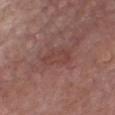Clinical impression: The lesion was tiled from a total-body skin photograph and was not biopsied. Clinical summary: From the front of the torso. This is a white-light tile. A roughly 15 mm field-of-view crop from a total-body skin photograph. The total-body-photography lesion software estimated two-axis asymmetry of about 0.25. The software also gave border irregularity of about 3 on a 0–10 scale and internal color variation of about 1.5 on a 0–10 scale. The analysis additionally found a classifier nevus-likeness of about 0/100 and lesion-presence confidence of about 100/100. The lesion's longest dimension is about 4 mm. The subject is a male roughly 65 years of age.